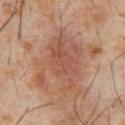| feature | finding |
|---|---|
| notes | total-body-photography surveillance lesion; no biopsy |
| tile lighting | cross-polarized illumination |
| imaging modality | ~15 mm tile from a whole-body skin photo |
| lesion diameter | ≈8.5 mm |
| subject | male, aged 58 to 62 |
| location | the abdomen |